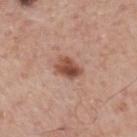Background: A close-up tile cropped from a whole-body skin photograph, about 15 mm across. The recorded lesion diameter is about 3.5 mm. The lesion is located on the mid back. A male subject, about 75 years old.